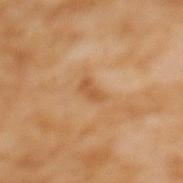biopsy status: catalogued during a skin exam; not biopsied | imaging modality: 15 mm crop, total-body photography | tile lighting: cross-polarized illumination | diameter: ~3 mm (longest diameter) | body site: the mid back | subject: female, about 55 years old.